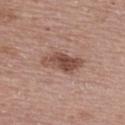Assessment:
The lesion was photographed on a routine skin check and not biopsied; there is no pathology result.
Clinical summary:
The patient is a female in their mid- to late 60s. Imaged with white-light lighting. Located on the upper back. An algorithmic analysis of the crop reported a mean CIELAB color near L≈48 a*≈21 b*≈25, roughly 12 lightness units darker than nearby skin, and a normalized lesion–skin contrast near 9. And it measured internal color variation of about 4.5 on a 0–10 scale and peripheral color asymmetry of about 1.5. A 15 mm crop from a total-body photograph taken for skin-cancer surveillance.